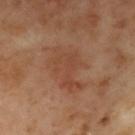Impression:
This lesion was catalogued during total-body skin photography and was not selected for biopsy.
Image and clinical context:
Located on the upper back. A close-up tile cropped from a whole-body skin photograph, about 15 mm across. Measured at roughly 5.5 mm in maximum diameter. A female subject approximately 60 years of age. This is a cross-polarized tile.